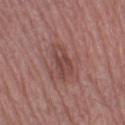No biopsy was performed on this lesion — it was imaged during a full skin examination and was not determined to be concerning. Measured at roughly 4 mm in maximum diameter. A region of skin cropped from a whole-body photographic capture, roughly 15 mm wide. The lesion is located on the left lower leg. Captured under white-light illumination. A female subject, in their mid-50s.